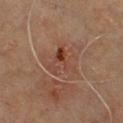notes=total-body-photography surveillance lesion; no biopsy
patient=male, aged 68–72
image=~15 mm tile from a whole-body skin photo
illumination=cross-polarized
TBP lesion metrics=internal color variation of about 9.5 on a 0–10 scale and radial color variation of about 3.5; lesion-presence confidence of about 100/100
body site=the chest